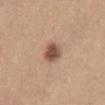| key | value |
|---|---|
| patient | female, roughly 25 years of age |
| body site | the lower back |
| image | ~15 mm tile from a whole-body skin photo |